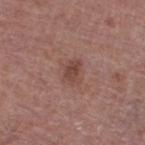Impression: No biopsy was performed on this lesion — it was imaged during a full skin examination and was not determined to be concerning. Image and clinical context: A roughly 15 mm field-of-view crop from a total-body skin photograph. Located on the right thigh. Measured at roughly 2.5 mm in maximum diameter. A male patient aged 73 to 77. The tile uses white-light illumination.